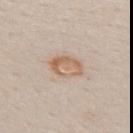Findings:
- biopsy status · no biopsy performed (imaged during a skin exam)
- anatomic site · the upper back
- acquisition · ~15 mm tile from a whole-body skin photo
- patient · female, in their mid- to late 20s